A female subject approximately 35 years of age, this image is a 15 mm lesion crop taken from a total-body photograph, from the abdomen — 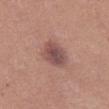Approximately 3.5 mm at its widest. Captured under white-light illumination. An algorithmic analysis of the crop reported an area of roughly 8.5 mm², a shape eccentricity near 0.5, and two-axis asymmetry of about 0.15. It also reported a within-lesion color-variation index near 4/10 and peripheral color asymmetry of about 1. The analysis additionally found a lesion-detection confidence of about 100/100. Histopathologically confirmed as a dysplastic (Clark) nevus.